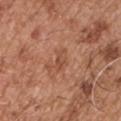Recorded during total-body skin imaging; not selected for excision or biopsy.
About 3 mm across.
From the chest.
This is a white-light tile.
A male patient, aged 53–57.
A 15 mm crop from a total-body photograph taken for skin-cancer surveillance.